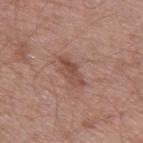{"biopsy_status": "not biopsied; imaged during a skin examination", "lesion_size": {"long_diameter_mm_approx": 4.0}, "automated_metrics": {"area_mm2_approx": 5.0, "eccentricity": 0.9, "shape_asymmetry": 0.4, "border_irregularity_0_10": 5.0, "color_variation_0_10": 2.5, "peripheral_color_asymmetry": 1.0, "nevus_likeness_0_100": 0, "lesion_detection_confidence_0_100": 100}, "image": {"source": "total-body photography crop", "field_of_view_mm": 15}, "patient": {"sex": "male", "age_approx": 60}, "site": "right thigh", "lighting": "white-light"}